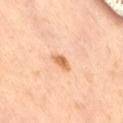The lesion was tiled from a total-body skin photograph and was not biopsied.
The lesion is located on the right thigh.
A female subject in their mid- to late 50s.
A 15 mm close-up extracted from a 3D total-body photography capture.
The lesion-visualizer software estimated a footprint of about 3 mm², an outline eccentricity of about 0.75 (0 = round, 1 = elongated), and two-axis asymmetry of about 0.3. The software also gave a border-irregularity index near 2.5/10, internal color variation of about 2.5 on a 0–10 scale, and a peripheral color-asymmetry measure near 1. It also reported an automated nevus-likeness rating near 95 out of 100 and a lesion-detection confidence of about 100/100.